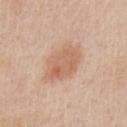notes = imaged on a skin check; not biopsied | size = ~5.5 mm (longest diameter) | automated lesion analysis = an area of roughly 14 mm², an eccentricity of roughly 0.75, and a symmetry-axis asymmetry near 0.15; peripheral color asymmetry of about 1; a detector confidence of about 100 out of 100 that the crop contains a lesion | site = the chest | illumination = white-light illumination | subject = male, about 60 years old | imaging modality = ~15 mm crop, total-body skin-cancer survey.